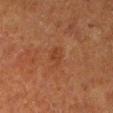Impression:
No biopsy was performed on this lesion — it was imaged during a full skin examination and was not determined to be concerning.
Clinical summary:
The tile uses cross-polarized illumination. Located on the right upper arm. A region of skin cropped from a whole-body photographic capture, roughly 15 mm wide. The recorded lesion diameter is about 2.5 mm. A female subject aged approximately 50. An algorithmic analysis of the crop reported an average lesion color of about L≈33 a*≈21 b*≈30 (CIELAB), roughly 5 lightness units darker than nearby skin, and a lesion-to-skin contrast of about 5 (normalized; higher = more distinct).